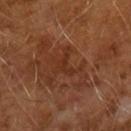notes=imaged on a skin check; not biopsied | automated metrics=a classifier nevus-likeness of about 0/100 and a lesion-detection confidence of about 100/100 | patient=male, in their mid-60s | lighting=cross-polarized illumination | image source=~15 mm tile from a whole-body skin photo.